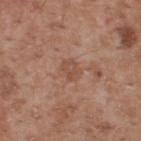This lesion was catalogued during total-body skin photography and was not selected for biopsy.
A male subject about 55 years old.
Measured at roughly 2.5 mm in maximum diameter.
The lesion is located on the upper back.
Imaged with white-light lighting.
A 15 mm close-up extracted from a 3D total-body photography capture.
Automated image analysis of the tile measured an outline eccentricity of about 0.6 (0 = round, 1 = elongated). The software also gave a mean CIELAB color near L≈50 a*≈21 b*≈29, a lesion–skin lightness drop of about 7, and a lesion-to-skin contrast of about 5 (normalized; higher = more distinct).